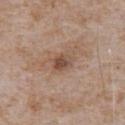Assessment:
The lesion was tiled from a total-body skin photograph and was not biopsied.
Clinical summary:
On the abdomen. The subject is a male aged 63–67. A 15 mm close-up tile from a total-body photography series done for melanoma screening.